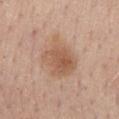Clinical impression:
The lesion was tiled from a total-body skin photograph and was not biopsied.
Clinical summary:
About 5 mm across. The subject is a female in their mid- to late 60s. From the back. This image is a 15 mm lesion crop taken from a total-body photograph.